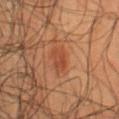No biopsy was performed on this lesion — it was imaged during a full skin examination and was not determined to be concerning. From the lower back. A close-up tile cropped from a whole-body skin photograph, about 15 mm across. An algorithmic analysis of the crop reported a lesion area of about 5.5 mm², a shape eccentricity near 0.7, and a symmetry-axis asymmetry near 0.2. The analysis additionally found an average lesion color of about L≈35 a*≈21 b*≈28 (CIELAB), a lesion–skin lightness drop of about 6, and a normalized lesion–skin contrast near 5.5. The software also gave a classifier nevus-likeness of about 50/100 and a lesion-detection confidence of about 100/100. Captured under cross-polarized illumination. A male patient, aged approximately 50.